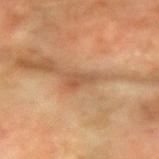| key | value |
|---|---|
| biopsy status | catalogued during a skin exam; not biopsied |
| body site | the right forearm |
| acquisition | total-body-photography crop, ~15 mm field of view |
| lighting | cross-polarized |
| patient | female, about 55 years old |